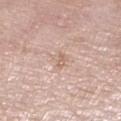{"biopsy_status": "not biopsied; imaged during a skin examination", "lesion_size": {"long_diameter_mm_approx": 2.5}, "image": {"source": "total-body photography crop", "field_of_view_mm": 15}, "lighting": "white-light", "patient": {"sex": "female", "age_approx": 75}, "site": "right lower leg"}